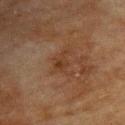Notes:
* notes — no biopsy performed (imaged during a skin exam)
* subject — female, aged 78–82
* acquisition — ~15 mm crop, total-body skin-cancer survey
* body site — the upper back